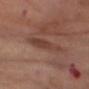biopsy status: no biopsy performed (imaged during a skin exam) | patient: female, about 55 years old | site: the right thigh | lesion diameter: about 5 mm | illumination: cross-polarized | acquisition: 15 mm crop, total-body photography | TBP lesion metrics: a mean CIELAB color near L≈42 a*≈20 b*≈26, a lesion–skin lightness drop of about 7, and a normalized border contrast of about 5.5; a border-irregularity index near 3/10, a color-variation rating of about 3.5/10, and a peripheral color-asymmetry measure near 1.